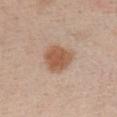Findings:
* workup · imaged on a skin check; not biopsied